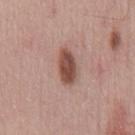{"image": {"source": "total-body photography crop", "field_of_view_mm": 15}, "patient": {"sex": "male", "age_approx": 45}, "lesion_size": {"long_diameter_mm_approx": 4.0}, "site": "chest", "lighting": "white-light"}